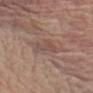| key | value |
|---|---|
| notes | catalogued during a skin exam; not biopsied |
| tile lighting | white-light illumination |
| image | 15 mm crop, total-body photography |
| body site | the left upper arm |
| subject | male, approximately 65 years of age |